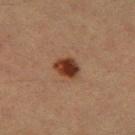This lesion was catalogued during total-body skin photography and was not selected for biopsy.
A close-up tile cropped from a whole-body skin photograph, about 15 mm across.
Captured under cross-polarized illumination.
The lesion is located on the left thigh.
Approximately 3 mm at its widest.
Automated tile analysis of the lesion measured a footprint of about 6 mm², an outline eccentricity of about 0.6 (0 = round, 1 = elongated), and two-axis asymmetry of about 0.15. And it measured an average lesion color of about L≈29 a*≈20 b*≈26 (CIELAB) and a normalized lesion–skin contrast near 12.5. And it measured a border-irregularity rating of about 1.5/10, a color-variation rating of about 4.5/10, and radial color variation of about 1.5.
A male subject, aged 38–42.